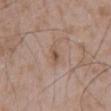{
  "biopsy_status": "not biopsied; imaged during a skin examination",
  "image": {
    "source": "total-body photography crop",
    "field_of_view_mm": 15
  },
  "patient": {
    "sex": "male",
    "age_approx": 50
  },
  "site": "back"
}